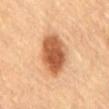{"biopsy_status": "not biopsied; imaged during a skin examination", "patient": {"sex": "male", "age_approx": "85+"}, "lesion_size": {"long_diameter_mm_approx": 6.0}, "image": {"source": "total-body photography crop", "field_of_view_mm": 15}, "site": "chest"}